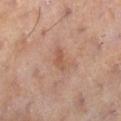Impression:
The lesion was tiled from a total-body skin photograph and was not biopsied.
Clinical summary:
The lesion is on the right lower leg. This is a cross-polarized tile. About 2.5 mm across. A female patient approximately 50 years of age. A 15 mm crop from a total-body photograph taken for skin-cancer surveillance.